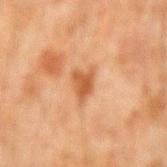Clinical summary:
The patient is a male aged 43 to 47. Captured under cross-polarized illumination. A lesion tile, about 15 mm wide, cut from a 3D total-body photograph. About 3 mm across. From the right forearm.